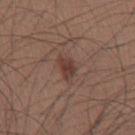<case>
  <biopsy_status>not biopsied; imaged during a skin examination</biopsy_status>
  <site>back</site>
  <lesion_size>
    <long_diameter_mm_approx>3.0</long_diameter_mm_approx>
  </lesion_size>
  <lighting>white-light</lighting>
  <patient>
    <sex>male</sex>
    <age_approx>35</age_approx>
  </patient>
  <image>
    <source>total-body photography crop</source>
    <field_of_view_mm>15</field_of_view_mm>
  </image>
</case>